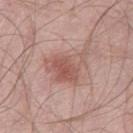Clinical impression: Recorded during total-body skin imaging; not selected for excision or biopsy. Context: A close-up tile cropped from a whole-body skin photograph, about 15 mm across. Imaged with white-light lighting. Automated tile analysis of the lesion measured a border-irregularity index near 5/10, internal color variation of about 5 on a 0–10 scale, and a peripheral color-asymmetry measure near 1.5. The analysis additionally found a nevus-likeness score of about 45/100 and lesion-presence confidence of about 100/100. A male patient, in their mid- to late 50s. From the left thigh.